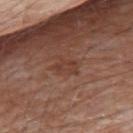imaging modality = 15 mm crop, total-body photography
lesion size = ~3 mm (longest diameter)
lighting = white-light
site = the upper back
patient = male, roughly 80 years of age